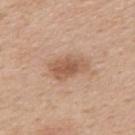The lesion was tiled from a total-body skin photograph and was not biopsied.
A close-up tile cropped from a whole-body skin photograph, about 15 mm across.
A male subject, about 50 years old.
Located on the mid back.